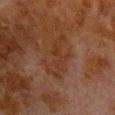follow-up: imaged on a skin check; not biopsied | lighting: cross-polarized | location: the chest | size: ≈6 mm | image-analysis metrics: a footprint of about 9 mm², an outline eccentricity of about 0.95 (0 = round, 1 = elongated), and two-axis asymmetry of about 0.5; a mean CIELAB color near L≈27 a*≈18 b*≈25, roughly 4 lightness units darker than nearby skin, and a normalized lesion–skin contrast near 5.5 | subject: male, about 80 years old | image: ~15 mm tile from a whole-body skin photo.